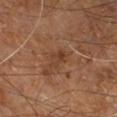No biopsy was performed on this lesion — it was imaged during a full skin examination and was not determined to be concerning. A male patient, roughly 60 years of age. On the right leg. This image is a 15 mm lesion crop taken from a total-body photograph.